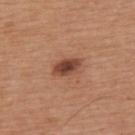Findings:
- workup — imaged on a skin check; not biopsied
- body site — the back
- lesion size — ≈3.5 mm
- patient — male, in their mid-60s
- acquisition — total-body-photography crop, ~15 mm field of view